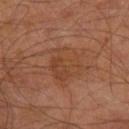Case summary:
- follow-up — no biopsy performed (imaged during a skin exam)
- subject — male, approximately 60 years of age
- anatomic site — the leg
- diameter — about 5 mm
- automated metrics — a lesion area of about 14 mm², a shape eccentricity near 0.45, and two-axis asymmetry of about 0.25
- illumination — cross-polarized
- imaging modality — ~15 mm crop, total-body skin-cancer survey